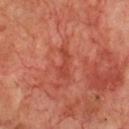workup = catalogued during a skin exam; not biopsied | acquisition = ~15 mm crop, total-body skin-cancer survey | tile lighting = cross-polarized illumination | patient = male, in their 70s | size = ≈3.5 mm | body site = the chest | TBP lesion metrics = a lesion area of about 4 mm² and a symmetry-axis asymmetry near 0.55; a border-irregularity rating of about 6.5/10, a color-variation rating of about 0.5/10, and peripheral color asymmetry of about 0; a classifier nevus-likeness of about 0/100 and a lesion-detection confidence of about 95/100.